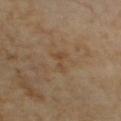Findings:
* workup — catalogued during a skin exam; not biopsied
* image — ~15 mm tile from a whole-body skin photo
* automated metrics — an area of roughly 3 mm²; a border-irregularity index near 7/10, a color-variation rating of about 0/10, and radial color variation of about 0
* anatomic site — the chest
* lesion size — about 2.5 mm
* subject — female